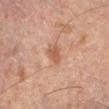Captured during whole-body skin photography for melanoma surveillance; the lesion was not biopsied. The patient is a male in their mid-60s. The lesion is located on the left lower leg. Automated image analysis of the tile measured a shape eccentricity near 0.75 and two-axis asymmetry of about 0.3. It also reported an average lesion color of about L≈48 a*≈20 b*≈29 (CIELAB), about 8 CIELAB-L* units darker than the surrounding skin, and a lesion-to-skin contrast of about 6.5 (normalized; higher = more distinct). The software also gave a nevus-likeness score of about 0/100 and a detector confidence of about 100 out of 100 that the crop contains a lesion. Captured under cross-polarized illumination. The recorded lesion diameter is about 2.5 mm. A 15 mm close-up extracted from a 3D total-body photography capture.